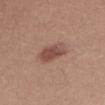Assessment:
The lesion was tiled from a total-body skin photograph and was not biopsied.
Background:
Imaged with white-light lighting. The total-body-photography lesion software estimated a mean CIELAB color near L≈49 a*≈21 b*≈25, a lesion–skin lightness drop of about 11, and a lesion-to-skin contrast of about 8 (normalized; higher = more distinct). And it measured internal color variation of about 3.5 on a 0–10 scale and a peripheral color-asymmetry measure near 1. The patient is a female aged 43–47. On the right thigh. About 3.5 mm across. A region of skin cropped from a whole-body photographic capture, roughly 15 mm wide.